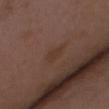Findings:
– workup · no biopsy performed (imaged during a skin exam)
– illumination · white-light illumination
– size · ~2.5 mm (longest diameter)
– acquisition · 15 mm crop, total-body photography
– subject · female, in their mid-30s
– location · the chest
– image-analysis metrics · a footprint of about 3.5 mm²; a border-irregularity rating of about 2.5/10, a color-variation rating of about 1/10, and a peripheral color-asymmetry measure near 0.5